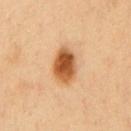Acquisition and patient details: A male patient, in their 50s. This is a cross-polarized tile. This image is a 15 mm lesion crop taken from a total-body photograph. The lesion is located on the chest. Automated image analysis of the tile measured a lesion area of about 10 mm², a shape eccentricity near 0.7, and a shape-asymmetry score of about 0.15 (0 = symmetric). The analysis additionally found a border-irregularity rating of about 1.5/10, internal color variation of about 6.5 on a 0–10 scale, and a peripheral color-asymmetry measure near 2. The analysis additionally found an automated nevus-likeness rating near 100 out of 100 and a detector confidence of about 100 out of 100 that the crop contains a lesion.